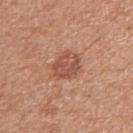Q: Is there a histopathology result?
A: imaged on a skin check; not biopsied
Q: Where on the body is the lesion?
A: the right upper arm
Q: Patient demographics?
A: female, about 50 years old
Q: What kind of image is this?
A: 15 mm crop, total-body photography